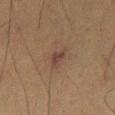Clinical impression: Recorded during total-body skin imaging; not selected for excision or biopsy. Clinical summary: The lesion is on the left thigh. Measured at roughly 2.5 mm in maximum diameter. Captured under cross-polarized illumination. A roughly 15 mm field-of-view crop from a total-body skin photograph. A male subject, roughly 50 years of age.